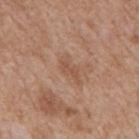Impression:
Part of a total-body skin-imaging series; this lesion was reviewed on a skin check and was not flagged for biopsy.
Clinical summary:
The lesion is located on the mid back. A 15 mm crop from a total-body photograph taken for skin-cancer surveillance. The patient is a male aged 63–67.